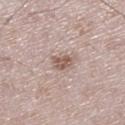The lesion was tiled from a total-body skin photograph and was not biopsied.